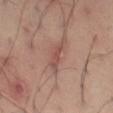{
  "biopsy_status": "not biopsied; imaged during a skin examination",
  "image": {
    "source": "total-body photography crop",
    "field_of_view_mm": 15
  },
  "lesion_size": {
    "long_diameter_mm_approx": 4.0
  },
  "patient": {
    "sex": "male",
    "age_approx": 40
  },
  "lighting": "cross-polarized",
  "site": "left thigh"
}